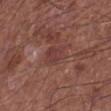Q: Is there a histopathology result?
A: no biopsy performed (imaged during a skin exam)
Q: How was the tile lit?
A: white-light illumination
Q: How was this image acquired?
A: ~15 mm crop, total-body skin-cancer survey
Q: Lesion size?
A: ≈3.5 mm
Q: Where on the body is the lesion?
A: the left lower leg
Q: What are the patient's age and sex?
A: male, roughly 65 years of age
Q: What did automated image analysis measure?
A: a lesion area of about 7 mm², an eccentricity of roughly 0.75, and a symmetry-axis asymmetry near 0.3; a mean CIELAB color near L≈39 a*≈23 b*≈22, roughly 6 lightness units darker than nearby skin, and a normalized lesion–skin contrast near 5.5; border irregularity of about 3 on a 0–10 scale, a color-variation rating of about 2/10, and peripheral color asymmetry of about 0.5; lesion-presence confidence of about 95/100